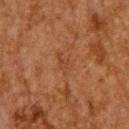| field | value |
|---|---|
| follow-up | imaged on a skin check; not biopsied |
| TBP lesion metrics | a lesion area of about 2.5 mm², an eccentricity of roughly 0.8, and two-axis asymmetry of about 0.6 |
| image source | ~15 mm crop, total-body skin-cancer survey |
| location | the upper back |
| patient | male, aged 58–62 |